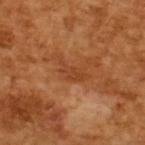Q: Is there a histopathology result?
A: no biopsy performed (imaged during a skin exam)